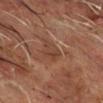biopsy_status: not biopsied; imaged during a skin examination
image:
  source: total-body photography crop
  field_of_view_mm: 15
lighting: cross-polarized
lesion_size:
  long_diameter_mm_approx: 3.0
patient:
  sex: male
  age_approx: 70
site: head or neck